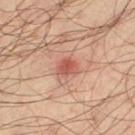Notes:
- follow-up · catalogued during a skin exam; not biopsied
- patient · male, approximately 35 years of age
- imaging modality · total-body-photography crop, ~15 mm field of view
- anatomic site · the left thigh